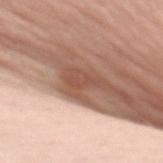Findings:
- notes: imaged on a skin check; not biopsied
- subject: female, in their 50s
- illumination: white-light
- image: total-body-photography crop, ~15 mm field of view
- location: the upper back